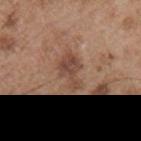location: the front of the torso
subject: male, about 55 years old
image: total-body-photography crop, ~15 mm field of view